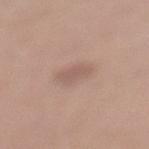workup — imaged on a skin check; not biopsied
size — about 3 mm
image source — 15 mm crop, total-body photography
subject — female, aged around 50
tile lighting — white-light
body site — the left lower leg